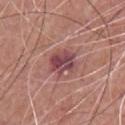This lesion was catalogued during total-body skin photography and was not selected for biopsy. A male patient, aged 53–57. The recorded lesion diameter is about 3.5 mm. Captured under white-light illumination. On the front of the torso. Cropped from a whole-body photographic skin survey; the tile spans about 15 mm. Automated tile analysis of the lesion measured an area of roughly 8.5 mm², an eccentricity of roughly 0.5, and a symmetry-axis asymmetry near 0.2. It also reported an average lesion color of about L≈46 a*≈26 b*≈18 (CIELAB), about 12 CIELAB-L* units darker than the surrounding skin, and a normalized lesion–skin contrast near 9.5. It also reported lesion-presence confidence of about 100/100.